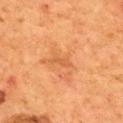<record>
<biopsy_status>not biopsied; imaged during a skin examination</biopsy_status>
<site>back</site>
<image>
  <source>total-body photography crop</source>
  <field_of_view_mm>15</field_of_view_mm>
</image>
<lighting>cross-polarized</lighting>
<lesion_size>
  <long_diameter_mm_approx>2.5</long_diameter_mm_approx>
</lesion_size>
<automated_metrics>
  <border_irregularity_0_10>4.5</border_irregularity_0_10>
  <color_variation_0_10>0.0</color_variation_0_10>
  <peripheral_color_asymmetry>0.0</peripheral_color_asymmetry>
  <nevus_likeness_0_100>0</nevus_likeness_0_100>
  <lesion_detection_confidence_0_100>100</lesion_detection_confidence_0_100>
</automated_metrics>
<patient>
  <sex>male</sex>
  <age_approx>55</age_approx>
</patient>
</record>